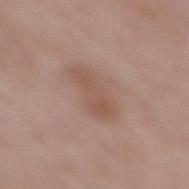This lesion was catalogued during total-body skin photography and was not selected for biopsy. A close-up tile cropped from a whole-body skin photograph, about 15 mm across. On the mid back. This is a white-light tile. A female subject, aged 68–72.